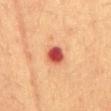Imaged during a routine full-body skin examination; the lesion was not biopsied and no histopathology is available.
Approximately 2.5 mm at its widest.
A close-up tile cropped from a whole-body skin photograph, about 15 mm across.
A male patient roughly 60 years of age.
From the abdomen.
This is a cross-polarized tile.